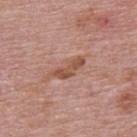| field | value |
|---|---|
| biopsy status | no biopsy performed (imaged during a skin exam) |
| automated lesion analysis | an area of roughly 5.5 mm² and a shape eccentricity near 0.95; a lesion color around L≈52 a*≈23 b*≈30 in CIELAB, a lesion–skin lightness drop of about 10, and a lesion-to-skin contrast of about 8 (normalized; higher = more distinct); a within-lesion color-variation index near 2/10 and a peripheral color-asymmetry measure near 0.5; a nevus-likeness score of about 0/100 |
| body site | the back |
| subject | male, aged around 75 |
| imaging modality | total-body-photography crop, ~15 mm field of view |
| diameter | ≈4.5 mm |
| tile lighting | white-light illumination |